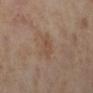biopsy_status: not biopsied; imaged during a skin examination
image:
  source: total-body photography crop
  field_of_view_mm: 15
automated_metrics:
  area_mm2_approx: 6.0
  eccentricity: 0.5
  shape_asymmetry: 0.3
  nevus_likeness_0_100: 0
  lesion_detection_confidence_0_100: 100
lighting: cross-polarized
site: left lower leg
lesion_size:
  long_diameter_mm_approx: 3.0
patient:
  sex: female
  age_approx: 55